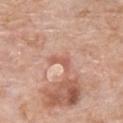Assessment:
The lesion was tiled from a total-body skin photograph and was not biopsied.
Background:
The lesion is located on the chest. This is a white-light tile. A male patient in their 60s. A close-up tile cropped from a whole-body skin photograph, about 15 mm across.